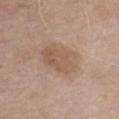notes = no biopsy performed (imaged during a skin exam)
subject = male, aged 73–77
location = the front of the torso
lesion diameter = ~5 mm (longest diameter)
imaging modality = ~15 mm tile from a whole-body skin photo
tile lighting = white-light illumination